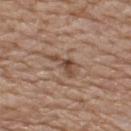{"biopsy_status": "not biopsied; imaged during a skin examination", "automated_metrics": {"cielab_L": 46, "cielab_a": 18, "cielab_b": 28, "vs_skin_darker_L": 11.0, "vs_skin_contrast_norm": 8.0, "nevus_likeness_0_100": 10, "lesion_detection_confidence_0_100": 90}, "patient": {"sex": "male", "age_approx": 80}, "site": "upper back", "image": {"source": "total-body photography crop", "field_of_view_mm": 15}, "lighting": "white-light", "lesion_size": {"long_diameter_mm_approx": 3.5}}